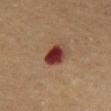Located on the chest.
Imaged with cross-polarized lighting.
A male subject aged around 60.
Cropped from a whole-body photographic skin survey; the tile spans about 15 mm.